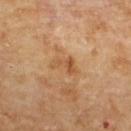No biopsy was performed on this lesion — it was imaged during a full skin examination and was not determined to be concerning. The lesion is on the upper back. Longest diameter approximately 3.5 mm. A male subject aged 83 or over. This image is a 15 mm lesion crop taken from a total-body photograph.